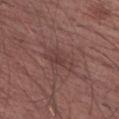Captured during whole-body skin photography for melanoma surveillance; the lesion was not biopsied. A male subject, about 65 years old. A 15 mm crop from a total-body photograph taken for skin-cancer surveillance. Captured under white-light illumination. The total-body-photography lesion software estimated an area of roughly 7 mm², an eccentricity of roughly 0.75, and a symmetry-axis asymmetry near 0.3. About 4 mm across. The lesion is located on the right upper arm.